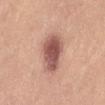Impression:
The lesion was tiled from a total-body skin photograph and was not biopsied.
Background:
The recorded lesion diameter is about 5.5 mm. A 15 mm crop from a total-body photograph taken for skin-cancer surveillance. From the front of the torso. The total-body-photography lesion software estimated an area of roughly 14 mm², a shape eccentricity near 0.8, and two-axis asymmetry of about 0.2. It also reported border irregularity of about 2 on a 0–10 scale and internal color variation of about 5 on a 0–10 scale. A female patient roughly 25 years of age. The tile uses white-light illumination.